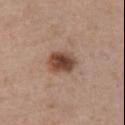Notes:
* follow-up: total-body-photography surveillance lesion; no biopsy